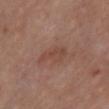Q: Was this lesion biopsied?
A: no biopsy performed (imaged during a skin exam)